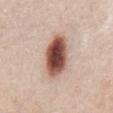follow-up: total-body-photography surveillance lesion; no biopsy
image-analysis metrics: an area of roughly 15 mm², an outline eccentricity of about 0.8 (0 = round, 1 = elongated), and a shape-asymmetry score of about 0.1 (0 = symmetric); border irregularity of about 1.5 on a 0–10 scale, a color-variation rating of about 9/10, and a peripheral color-asymmetry measure near 2.5
imaging modality: ~15 mm tile from a whole-body skin photo
size: about 6 mm
illumination: white-light illumination
patient: male, aged around 60
site: the chest